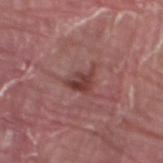Part of a total-body skin-imaging series; this lesion was reviewed on a skin check and was not flagged for biopsy.
A male patient about 40 years old.
A close-up tile cropped from a whole-body skin photograph, about 15 mm across.
Located on the right thigh.
The lesion's longest dimension is about 3.5 mm.
Captured under white-light illumination.